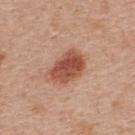<tbp_lesion>
<patient>
  <sex>male</sex>
  <age_approx>55</age_approx>
</patient>
<lesion_size>
  <long_diameter_mm_approx>4.5</long_diameter_mm_approx>
</lesion_size>
<lighting>white-light</lighting>
<image>
  <source>total-body photography crop</source>
  <field_of_view_mm>15</field_of_view_mm>
</image>
<site>back</site>
</tbp_lesion>